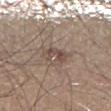follow-up: no biopsy performed (imaged during a skin exam); patient: male, aged around 45; illumination: white-light; anatomic site: the right lower leg; image source: ~15 mm crop, total-body skin-cancer survey; diameter: about 3 mm.